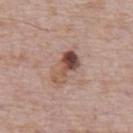Q: Was a biopsy performed?
A: no biopsy performed (imaged during a skin exam)
Q: What lighting was used for the tile?
A: white-light illumination
Q: Lesion size?
A: ≈4.5 mm
Q: What is the anatomic site?
A: the abdomen
Q: How was this image acquired?
A: 15 mm crop, total-body photography
Q: Patient demographics?
A: male, approximately 70 years of age